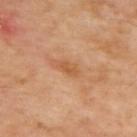The lesion was photographed on a routine skin check and not biopsied; there is no pathology result. On the back. An algorithmic analysis of the crop reported a mean CIELAB color near L≈57 a*≈24 b*≈40, about 8 CIELAB-L* units darker than the surrounding skin, and a normalized lesion–skin contrast near 6. The software also gave a border-irregularity index near 3/10 and internal color variation of about 1 on a 0–10 scale. And it measured a classifier nevus-likeness of about 0/100. About 3 mm across. A lesion tile, about 15 mm wide, cut from a 3D total-body photograph.